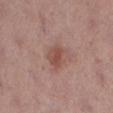Captured during whole-body skin photography for melanoma surveillance; the lesion was not biopsied.
On the left lower leg.
A 15 mm close-up extracted from a 3D total-body photography capture.
Measured at roughly 3 mm in maximum diameter.
A female patient about 40 years old.
Captured under white-light illumination.